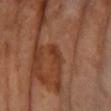Clinical impression: The lesion was photographed on a routine skin check and not biopsied; there is no pathology result. Context: A 15 mm crop from a total-body photograph taken for skin-cancer surveillance. Located on the left forearm. A female subject, aged around 65. Longest diameter approximately 3 mm.